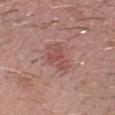Impression:
Imaged during a routine full-body skin examination; the lesion was not biopsied and no histopathology is available.
Background:
Cropped from a whole-body photographic skin survey; the tile spans about 15 mm. About 3.5 mm across. A male subject aged 28 to 32.